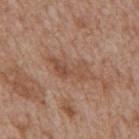<case>
<biopsy_status>not biopsied; imaged during a skin examination</biopsy_status>
<patient>
  <sex>male</sex>
  <age_approx>65</age_approx>
</patient>
<image>
  <source>total-body photography crop</source>
  <field_of_view_mm>15</field_of_view_mm>
</image>
<site>mid back</site>
<automated_metrics>
  <border_irregularity_0_10>7.0</border_irregularity_0_10>
</automated_metrics>
<lesion_size>
  <long_diameter_mm_approx>4.5</long_diameter_mm_approx>
</lesion_size>
</case>